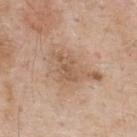{
  "automated_metrics": {
    "shape_asymmetry": 0.35,
    "nevus_likeness_0_100": 0
  },
  "image": {
    "source": "total-body photography crop",
    "field_of_view_mm": 15
  },
  "patient": {
    "sex": "male",
    "age_approx": 60
  },
  "site": "upper back",
  "lesion_size": {
    "long_diameter_mm_approx": 6.5
  },
  "lighting": "white-light"
}